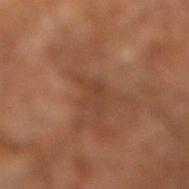Q: Was this lesion biopsied?
A: catalogued during a skin exam; not biopsied
Q: Automated lesion metrics?
A: an area of roughly 4.5 mm², an eccentricity of roughly 0.85, and two-axis asymmetry of about 0.5; border irregularity of about 6.5 on a 0–10 scale, internal color variation of about 1.5 on a 0–10 scale, and peripheral color asymmetry of about 0.5; a classifier nevus-likeness of about 0/100 and lesion-presence confidence of about 55/100
Q: How was the tile lit?
A: cross-polarized illumination
Q: Lesion location?
A: the right leg
Q: Lesion size?
A: about 4 mm
Q: What is the imaging modality?
A: 15 mm crop, total-body photography
Q: Patient demographics?
A: male, aged 58–62